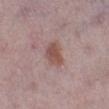Context: On the left lower leg. A female subject, aged 48–52. A 15 mm close-up extracted from a 3D total-body photography capture.